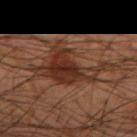notes: catalogued during a skin exam; not biopsied
TBP lesion metrics: a lesion area of about 20 mm², an eccentricity of roughly 0.85, and a shape-asymmetry score of about 0.5 (0 = symmetric); an average lesion color of about L≈25 a*≈17 b*≈23 (CIELAB), roughly 8 lightness units darker than nearby skin, and a normalized border contrast of about 8.5; a border-irregularity rating of about 8/10, internal color variation of about 5 on a 0–10 scale, and peripheral color asymmetry of about 1.5
subject: male, in their 50s
anatomic site: the arm
tile lighting: cross-polarized
lesion size: about 9 mm
imaging modality: total-body-photography crop, ~15 mm field of view